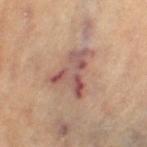Q: Was this lesion biopsied?
A: catalogued during a skin exam; not biopsied
Q: What are the patient's age and sex?
A: female, roughly 65 years of age
Q: What is the anatomic site?
A: the left thigh
Q: What kind of image is this?
A: total-body-photography crop, ~15 mm field of view